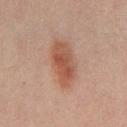The lesion was tiled from a total-body skin photograph and was not biopsied.
From the front of the torso.
Captured under cross-polarized illumination.
The lesion-visualizer software estimated a within-lesion color-variation index near 4/10 and radial color variation of about 1.5.
Measured at roughly 5.5 mm in maximum diameter.
This image is a 15 mm lesion crop taken from a total-body photograph.
The subject is a male in their 30s.